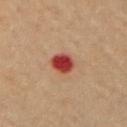Findings:
– workup — total-body-photography surveillance lesion; no biopsy
– subject — male, aged approximately 55
– image — 15 mm crop, total-body photography
– size — about 3 mm
– site — the left arm